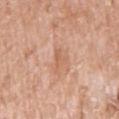This lesion was catalogued during total-body skin photography and was not selected for biopsy.
This image is a 15 mm lesion crop taken from a total-body photograph.
This is a white-light tile.
The lesion is located on the right upper arm.
Longest diameter approximately 3 mm.
The patient is a female roughly 85 years of age or older.